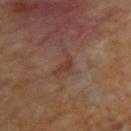follow-up: no biopsy performed (imaged during a skin exam) | location: the upper back | image: ~15 mm crop, total-body skin-cancer survey | diameter: about 2.5 mm | subject: about 60 years old.